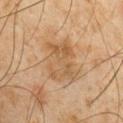  biopsy_status: not biopsied; imaged during a skin examination
  image:
    source: total-body photography crop
    field_of_view_mm: 15
  lighting: cross-polarized
  site: chest
  patient:
    sex: male
    age_approx: 60